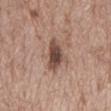{"biopsy_status": "not biopsied; imaged during a skin examination", "site": "mid back", "automated_metrics": {"border_irregularity_0_10": 4.0, "color_variation_0_10": 4.5, "peripheral_color_asymmetry": 1.0, "nevus_likeness_0_100": 50}, "image": {"source": "total-body photography crop", "field_of_view_mm": 15}, "lighting": "white-light", "patient": {"sex": "male", "age_approx": 70}, "lesion_size": {"long_diameter_mm_approx": 5.0}}